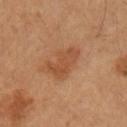Assessment:
The lesion was photographed on a routine skin check and not biopsied; there is no pathology result.
Context:
The patient is a male approximately 55 years of age. A roughly 15 mm field-of-view crop from a total-body skin photograph. Automated image analysis of the tile measured a lesion color around L≈45 a*≈22 b*≈33 in CIELAB and a normalized border contrast of about 6. It also reported border irregularity of about 4 on a 0–10 scale, a color-variation rating of about 2.5/10, and peripheral color asymmetry of about 1. The analysis additionally found a nevus-likeness score of about 15/100 and lesion-presence confidence of about 100/100. The lesion's longest dimension is about 4.5 mm. The lesion is located on the arm. Captured under cross-polarized illumination.